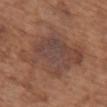biopsy status: no biopsy performed (imaged during a skin exam) | site: the upper back | lighting: white-light | patient: female, aged approximately 75 | image: total-body-photography crop, ~15 mm field of view | lesion size: about 8.5 mm | automated metrics: a lesion area of about 36 mm², an eccentricity of roughly 0.75, and a symmetry-axis asymmetry near 0.25; a lesion-detection confidence of about 100/100.